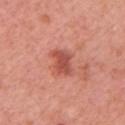<case>
<biopsy_status>not biopsied; imaged during a skin examination</biopsy_status>
<lighting>white-light</lighting>
<image>
  <source>total-body photography crop</source>
  <field_of_view_mm>15</field_of_view_mm>
</image>
<lesion_size>
  <long_diameter_mm_approx>3.0</long_diameter_mm_approx>
</lesion_size>
<patient>
  <sex>female</sex>
  <age_approx>55</age_approx>
</patient>
<site>arm</site>
</case>